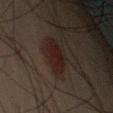workup: no biopsy performed (imaged during a skin exam)
acquisition: ~15 mm tile from a whole-body skin photo
location: the left upper arm
tile lighting: cross-polarized
patient: male, in their 50s
size: ~4 mm (longest diameter)